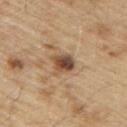Clinical summary:
A male subject aged approximately 70. The lesion's longest dimension is about 3 mm. A 15 mm close-up tile from a total-body photography series done for melanoma screening. Captured under white-light illumination. From the upper back.